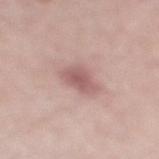Notes:
- workup: total-body-photography surveillance lesion; no biopsy
- site: the right lower leg
- subject: male, aged around 70
- illumination: white-light
- acquisition: 15 mm crop, total-body photography
- TBP lesion metrics: a lesion area of about 7 mm² and an outline eccentricity of about 0.4 (0 = round, 1 = elongated); a mean CIELAB color near L≈58 a*≈22 b*≈20 and roughly 11 lightness units darker than nearby skin; border irregularity of about 2.5 on a 0–10 scale, internal color variation of about 2.5 on a 0–10 scale, and peripheral color asymmetry of about 0.5; a detector confidence of about 100 out of 100 that the crop contains a lesion